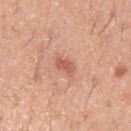The lesion is on the arm.
This is a white-light tile.
The patient is a male about 40 years old.
Approximately 2.5 mm at its widest.
Automated image analysis of the tile measured about 10 CIELAB-L* units darker than the surrounding skin.
A roughly 15 mm field-of-view crop from a total-body skin photograph.